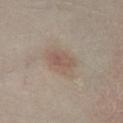follow-up=catalogued during a skin exam; not biopsied
site=the left lower leg
patient=male, aged 38–42
imaging modality=15 mm crop, total-body photography